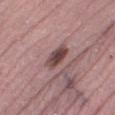follow-up — catalogued during a skin exam; not biopsied | subject — female, aged around 60 | TBP lesion metrics — a mean CIELAB color near L≈44 a*≈20 b*≈18, a lesion–skin lightness drop of about 14, and a lesion-to-skin contrast of about 10.5 (normalized; higher = more distinct); an automated nevus-likeness rating near 60 out of 100 and a lesion-detection confidence of about 100/100 | lesion diameter — ≈3.5 mm | illumination — white-light illumination | image — ~15 mm tile from a whole-body skin photo | anatomic site — the leg.